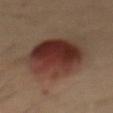notes: total-body-photography surveillance lesion; no biopsy | image source: 15 mm crop, total-body photography | anatomic site: the mid back | patient: male, about 30 years old.